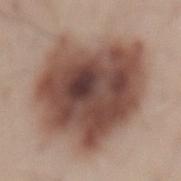• workup: total-body-photography surveillance lesion; no biopsy
• image source: 15 mm crop, total-body photography
• anatomic site: the mid back
• tile lighting: white-light
• subject: male, in their mid-50s
• size: about 9.5 mm